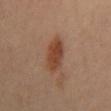Imaged during a routine full-body skin examination; the lesion was not biopsied and no histopathology is available. An algorithmic analysis of the crop reported a footprint of about 10 mm². The analysis additionally found a lesion–skin lightness drop of about 10. A 15 mm close-up tile from a total-body photography series done for melanoma screening. From the mid back. This is a cross-polarized tile. The recorded lesion diameter is about 5.5 mm. A female subject, aged approximately 35.